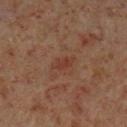| field | value |
|---|---|
| tile lighting | cross-polarized |
| lesion diameter | ~3 mm (longest diameter) |
| subject | male, aged approximately 60 |
| acquisition | 15 mm crop, total-body photography |
| body site | the left lower leg |